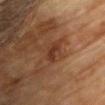Clinical impression: Captured during whole-body skin photography for melanoma surveillance; the lesion was not biopsied. Image and clinical context: A female subject approximately 80 years of age. Cropped from a total-body skin-imaging series; the visible field is about 15 mm. Approximately 2.5 mm at its widest. The lesion is on the chest. The tile uses cross-polarized illumination.